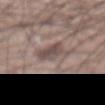follow-up: total-body-photography surveillance lesion; no biopsy
lighting: white-light
image: ~15 mm tile from a whole-body skin photo
patient: male, aged 58–62
lesion size: about 3.5 mm
anatomic site: the abdomen
TBP lesion metrics: a lesion color around L≈48 a*≈14 b*≈19 in CIELAB, roughly 10 lightness units darker than nearby skin, and a normalized border contrast of about 7.5; border irregularity of about 3.5 on a 0–10 scale and radial color variation of about 1; lesion-presence confidence of about 75/100